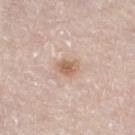Findings:
– patient — male, approximately 80 years of age
– site — the right thigh
– acquisition — 15 mm crop, total-body photography